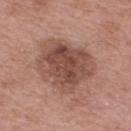notes: no biopsy performed (imaged during a skin exam) | lighting: white-light | location: the upper back | diameter: ≈7 mm | image: total-body-photography crop, ~15 mm field of view | patient: male, in their mid-60s.